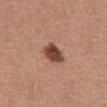Part of a total-body skin-imaging series; this lesion was reviewed on a skin check and was not flagged for biopsy.
The lesion is on the mid back.
Measured at roughly 3 mm in maximum diameter.
Imaged with white-light lighting.
Cropped from a total-body skin-imaging series; the visible field is about 15 mm.
The patient is a male aged 43–47.
The lesion-visualizer software estimated a lesion color around L≈45 a*≈23 b*≈28 in CIELAB, about 16 CIELAB-L* units darker than the surrounding skin, and a normalized border contrast of about 11.5. The software also gave an automated nevus-likeness rating near 95 out of 100 and a detector confidence of about 100 out of 100 that the crop contains a lesion.